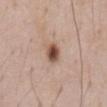Captured during whole-body skin photography for melanoma surveillance; the lesion was not biopsied. A roughly 15 mm field-of-view crop from a total-body skin photograph. The lesion is on the abdomen. A male patient about 60 years old.